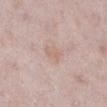Clinical summary: The lesion's longest dimension is about 3 mm. The tile uses white-light illumination. The subject is a female aged 43 to 47. A close-up tile cropped from a whole-body skin photograph, about 15 mm across. Automated tile analysis of the lesion measured a footprint of about 4 mm², a shape eccentricity near 0.75, and a symmetry-axis asymmetry near 0.2. The software also gave an automated nevus-likeness rating near 0 out of 100 and lesion-presence confidence of about 100/100. The lesion is on the right lower leg.